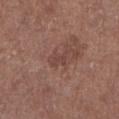{"patient": {"sex": "male", "age_approx": 75}, "lighting": "white-light", "lesion_size": {"long_diameter_mm_approx": 3.0}, "site": "left lower leg", "image": {"source": "total-body photography crop", "field_of_view_mm": 15}}